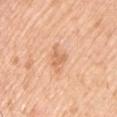The recorded lesion diameter is about 2 mm.
The lesion is on the left upper arm.
Cropped from a whole-body photographic skin survey; the tile spans about 15 mm.
The tile uses white-light illumination.
A male patient, in their mid- to late 50s.